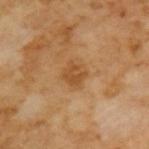{"biopsy_status": "not biopsied; imaged during a skin examination", "image": {"source": "total-body photography crop", "field_of_view_mm": 15}, "patient": {"sex": "male", "age_approx": 65}}